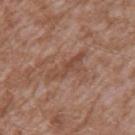Clinical impression: No biopsy was performed on this lesion — it was imaged during a full skin examination and was not determined to be concerning. Clinical summary: The patient is a male in their mid- to late 40s. The lesion is on the left upper arm. Measured at roughly 5 mm in maximum diameter. This image is a 15 mm lesion crop taken from a total-body photograph.